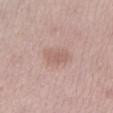Case summary:
* workup — no biopsy performed (imaged during a skin exam)
* site — the leg
* lesion size — ≈3.5 mm
* patient — female, aged approximately 30
* acquisition — 15 mm crop, total-body photography